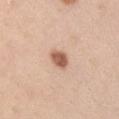Q: Is there a histopathology result?
A: no biopsy performed (imaged during a skin exam)
Q: Where on the body is the lesion?
A: the left upper arm
Q: How was this image acquired?
A: ~15 mm crop, total-body skin-cancer survey
Q: Automated lesion metrics?
A: an outline eccentricity of about 0.7 (0 = round, 1 = elongated); an average lesion color of about L≈58 a*≈22 b*≈31 (CIELAB), a lesion–skin lightness drop of about 16, and a lesion-to-skin contrast of about 10 (normalized; higher = more distinct); internal color variation of about 2.5 on a 0–10 scale and radial color variation of about 1; a classifier nevus-likeness of about 95/100 and lesion-presence confidence of about 100/100
Q: What is the lesion's diameter?
A: about 2.5 mm
Q: Who is the patient?
A: male, aged approximately 35